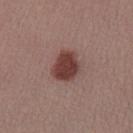Notes:
- follow-up: total-body-photography surveillance lesion; no biopsy
- tile lighting: white-light
- image source: ~15 mm crop, total-body skin-cancer survey
- patient: female, approximately 30 years of age
- site: the left forearm